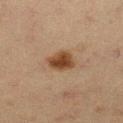follow-up: imaged on a skin check; not biopsied
subject: female, about 40 years old
lighting: cross-polarized illumination
imaging modality: total-body-photography crop, ~15 mm field of view
TBP lesion metrics: a lesion area of about 7.5 mm² and an eccentricity of roughly 0.5; border irregularity of about 2 on a 0–10 scale and internal color variation of about 3 on a 0–10 scale
size: ≈3.5 mm
location: the left lower leg